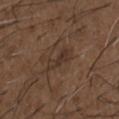No biopsy was performed on this lesion — it was imaged during a full skin examination and was not determined to be concerning. An algorithmic analysis of the crop reported a footprint of about 4.5 mm², an eccentricity of roughly 0.9, and two-axis asymmetry of about 0.5. And it measured a lesion color around L≈32 a*≈15 b*≈23 in CIELAB, a lesion–skin lightness drop of about 6, and a normalized lesion–skin contrast near 6.5. A lesion tile, about 15 mm wide, cut from a 3D total-body photograph. Captured under white-light illumination. The subject is a male in their 50s. Located on the chest.